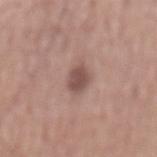Clinical impression:
Part of a total-body skin-imaging series; this lesion was reviewed on a skin check and was not flagged for biopsy.
Clinical summary:
Measured at roughly 3.5 mm in maximum diameter. From the mid back. A male subject aged approximately 55. A 15 mm close-up extracted from a 3D total-body photography capture. The tile uses white-light illumination.